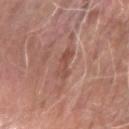Notes:
- anatomic site: the arm
- subject: male, approximately 80 years of age
- imaging modality: 15 mm crop, total-body photography
- lesion size: ~3.5 mm (longest diameter)